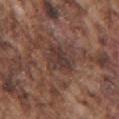biopsy status = total-body-photography surveillance lesion; no biopsy
subject = male, aged 73–77
image = ~15 mm crop, total-body skin-cancer survey
body site = the right upper arm